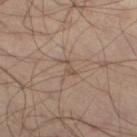Assessment: No biopsy was performed on this lesion — it was imaged during a full skin examination and was not determined to be concerning. Image and clinical context: Approximately 2.5 mm at its widest. Captured under cross-polarized illumination. A 15 mm crop from a total-body photograph taken for skin-cancer surveillance. A male subject aged 48 to 52. The lesion-visualizer software estimated internal color variation of about 0 on a 0–10 scale. Located on the lower back.